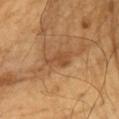Longest diameter approximately 3.5 mm.
The tile uses cross-polarized illumination.
From the front of the torso.
This image is a 15 mm lesion crop taken from a total-body photograph.
The patient is a male roughly 60 years of age.